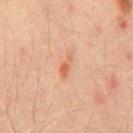No biopsy was performed on this lesion — it was imaged during a full skin examination and was not determined to be concerning. The subject is a male aged 43 to 47. Automated tile analysis of the lesion measured radial color variation of about 0. The analysis additionally found an automated nevus-likeness rating near 20 out of 100 and a detector confidence of about 100 out of 100 that the crop contains a lesion. The recorded lesion diameter is about 3 mm. The lesion is located on the chest. A lesion tile, about 15 mm wide, cut from a 3D total-body photograph.